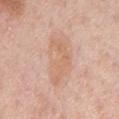Impression:
Recorded during total-body skin imaging; not selected for excision or biopsy.
Context:
From the chest. The subject is a male about 75 years old. This image is a 15 mm lesion crop taken from a total-body photograph.